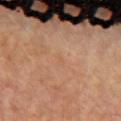The lesion was tiled from a total-body skin photograph and was not biopsied. The tile uses cross-polarized illumination. The patient is a female about 65 years old. An algorithmic analysis of the crop reported an average lesion color of about L≈56 a*≈20 b*≈33 (CIELAB), a lesion–skin lightness drop of about 3, and a normalized border contrast of about 3. It also reported border irregularity of about 3 on a 0–10 scale and peripheral color asymmetry of about 0. The analysis additionally found a nevus-likeness score of about 0/100. The lesion is on the chest. This image is a 15 mm lesion crop taken from a total-body photograph.